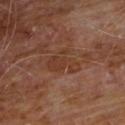| field | value |
|---|---|
| size | ≈4 mm |
| illumination | cross-polarized |
| image | 15 mm crop, total-body photography |
| location | the chest |
| patient | male, approximately 60 years of age |